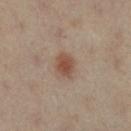Case summary:
* acquisition · total-body-photography crop, ~15 mm field of view
* tile lighting · cross-polarized
* site · the left leg
* patient · female, aged 38 to 42